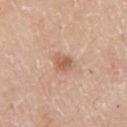<record>
<biopsy_status>not biopsied; imaged during a skin examination</biopsy_status>
<automated_metrics>
  <area_mm2_approx>4.5</area_mm2_approx>
  <eccentricity>0.55</eccentricity>
  <shape_asymmetry>0.3</shape_asymmetry>
</automated_metrics>
<patient>
  <sex>male</sex>
  <age_approx>60</age_approx>
</patient>
<lighting>white-light</lighting>
<site>chest</site>
<image>
  <source>total-body photography crop</source>
  <field_of_view_mm>15</field_of_view_mm>
</image>
</record>